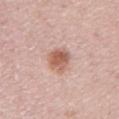The lesion was photographed on a routine skin check and not biopsied; there is no pathology result. The tile uses white-light illumination. Located on the left upper arm. Automated tile analysis of the lesion measured a footprint of about 7 mm² and a symmetry-axis asymmetry near 0.25. And it measured an average lesion color of about L≈59 a*≈22 b*≈28 (CIELAB), a lesion–skin lightness drop of about 12, and a lesion-to-skin contrast of about 8.5 (normalized; higher = more distinct). It also reported a border-irregularity index near 2/10, a color-variation rating of about 4/10, and peripheral color asymmetry of about 1.5. The analysis additionally found a classifier nevus-likeness of about 85/100 and a detector confidence of about 100 out of 100 that the crop contains a lesion. Measured at roughly 3.5 mm in maximum diameter. A female subject aged 48–52. A 15 mm close-up tile from a total-body photography series done for melanoma screening.